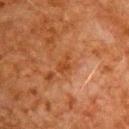A roughly 15 mm field-of-view crop from a total-body skin photograph. A male subject in their 80s. An algorithmic analysis of the crop reported a shape eccentricity near 0.8. The analysis additionally found a mean CIELAB color near L≈36 a*≈23 b*≈34 and about 6 CIELAB-L* units darker than the surrounding skin. The software also gave border irregularity of about 4 on a 0–10 scale, a within-lesion color-variation index near 1/10, and radial color variation of about 0.5. This is a cross-polarized tile. On the chest.